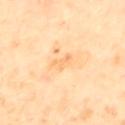Part of a total-body skin-imaging series; this lesion was reviewed on a skin check and was not flagged for biopsy.
A male patient aged approximately 65.
A 15 mm crop from a total-body photograph taken for skin-cancer surveillance.
Located on the back.